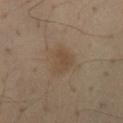follow-up: imaged on a skin check; not biopsied | tile lighting: cross-polarized illumination | patient: male, in their mid- to late 50s | image: 15 mm crop, total-body photography | diameter: ~3.5 mm (longest diameter) | body site: the back | TBP lesion metrics: an outline eccentricity of about 0.65 (0 = round, 1 = elongated) and two-axis asymmetry of about 0.3; a border-irregularity index near 3/10, internal color variation of about 2.5 on a 0–10 scale, and a peripheral color-asymmetry measure near 1; lesion-presence confidence of about 100/100.